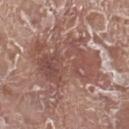Findings:
• notes · catalogued during a skin exam; not biopsied
• lighting · white-light
• location · the right lower leg
• size · ~8.5 mm (longest diameter)
• acquisition · ~15 mm tile from a whole-body skin photo
• subject · male, about 75 years old